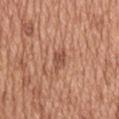Clinical impression: Recorded during total-body skin imaging; not selected for excision or biopsy. Clinical summary: Captured under white-light illumination. The recorded lesion diameter is about 2.5 mm. From the mid back. A male subject, roughly 65 years of age. A 15 mm close-up extracted from a 3D total-body photography capture.